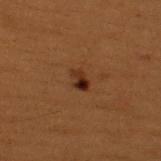Recorded during total-body skin imaging; not selected for excision or biopsy. About 2.5 mm across. A roughly 15 mm field-of-view crop from a total-body skin photograph. From the upper back. A male patient aged around 50. The tile uses cross-polarized illumination. The total-body-photography lesion software estimated an average lesion color of about L≈22 a*≈18 b*≈25 (CIELAB), roughly 9 lightness units darker than nearby skin, and a normalized border contrast of about 10. It also reported a classifier nevus-likeness of about 90/100 and a detector confidence of about 100 out of 100 that the crop contains a lesion.